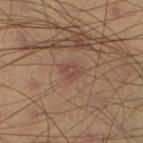<case>
  <biopsy_status>not biopsied; imaged during a skin examination</biopsy_status>
  <lesion_size>
    <long_diameter_mm_approx>2.5</long_diameter_mm_approx>
  </lesion_size>
  <image>
    <source>total-body photography crop</source>
    <field_of_view_mm>15</field_of_view_mm>
  </image>
  <patient>
    <sex>male</sex>
    <age_approx>40</age_approx>
  </patient>
  <site>right lower leg</site>
  <lighting>cross-polarized</lighting>
  <automated_metrics>
    <cielab_L>46</cielab_L>
    <cielab_a>19</cielab_a>
    <cielab_b>26</cielab_b>
    <vs_skin_darker_L>6.0</vs_skin_darker_L>
    <vs_skin_contrast_norm>5.0</vs_skin_contrast_norm>
    <border_irregularity_0_10>2.5</border_irregularity_0_10>
    <color_variation_0_10>3.0</color_variation_0_10>
    <peripheral_color_asymmetry>1.5</peripheral_color_asymmetry>
    <nevus_likeness_0_100>5</nevus_likeness_0_100>
    <lesion_detection_confidence_0_100>100</lesion_detection_confidence_0_100>
  </automated_metrics>
</case>